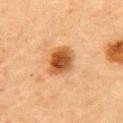Part of a total-body skin-imaging series; this lesion was reviewed on a skin check and was not flagged for biopsy.
On the back.
Approximately 3.5 mm at its widest.
The patient is a female roughly 60 years of age.
Captured under cross-polarized illumination.
Cropped from a whole-body photographic skin survey; the tile spans about 15 mm.